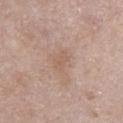Impression:
No biopsy was performed on this lesion — it was imaged during a full skin examination and was not determined to be concerning.
Image and clinical context:
From the chest. A 15 mm close-up tile from a total-body photography series done for melanoma screening. The patient is a male about 55 years old.